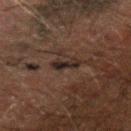No biopsy was performed on this lesion — it was imaged during a full skin examination and was not determined to be concerning. A lesion tile, about 15 mm wide, cut from a 3D total-body photograph. On the arm. A male subject, aged around 50. Captured under cross-polarized illumination.